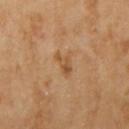workup: catalogued during a skin exam; not biopsied | automated metrics: a lesion area of about 3 mm², an outline eccentricity of about 0.8 (0 = round, 1 = elongated), and two-axis asymmetry of about 0.5; a mean CIELAB color near L≈53 a*≈21 b*≈38, a lesion–skin lightness drop of about 8, and a normalized lesion–skin contrast near 6.5; a border-irregularity index near 5/10, a color-variation rating of about 2.5/10, and a peripheral color-asymmetry measure near 0.5 | location: the right upper arm | patient: female, roughly 70 years of age | acquisition: 15 mm crop, total-body photography.